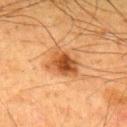biopsy status=catalogued during a skin exam; not biopsied
body site=the upper back
patient=male, aged 33–37
lesion diameter=≈4 mm
image=~15 mm crop, total-body skin-cancer survey
tile lighting=cross-polarized